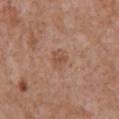Findings:
- workup: no biopsy performed (imaged during a skin exam)
- image source: ~15 mm tile from a whole-body skin photo
- anatomic site: the front of the torso
- patient: female, roughly 70 years of age